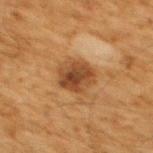follow-up: imaged on a skin check; not biopsied | subject: male, about 60 years old | anatomic site: the upper back | image source: ~15 mm tile from a whole-body skin photo | diameter: ≈4.5 mm | TBP lesion metrics: an outline eccentricity of about 0.65 (0 = round, 1 = elongated) and a symmetry-axis asymmetry near 0.15.